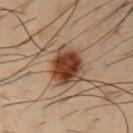biopsy status=no biopsy performed (imaged during a skin exam) | automated metrics=a lesion color around L≈40 a*≈22 b*≈30 in CIELAB, about 17 CIELAB-L* units darker than the surrounding skin, and a normalized border contrast of about 13; a border-irregularity index near 2/10 and a within-lesion color-variation index near 5.5/10 | lesion diameter=about 4 mm | lighting=cross-polarized illumination | patient=male, about 40 years old | location=the left upper arm | acquisition=total-body-photography crop, ~15 mm field of view.